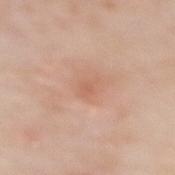This lesion was catalogued during total-body skin photography and was not selected for biopsy.